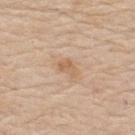Assessment: Recorded during total-body skin imaging; not selected for excision or biopsy. Clinical summary: The recorded lesion diameter is about 2.5 mm. A region of skin cropped from a whole-body photographic capture, roughly 15 mm wide. A male subject, aged 78 to 82. Located on the upper back.